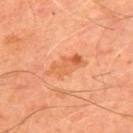Q: Where on the body is the lesion?
A: the upper back
Q: What did automated image analysis measure?
A: an outline eccentricity of about 0.9 (0 = round, 1 = elongated) and a shape-asymmetry score of about 0.3 (0 = symmetric); a mean CIELAB color near L≈63 a*≈29 b*≈43 and a normalized lesion–skin contrast near 6
Q: Who is the patient?
A: male, approximately 50 years of age
Q: What kind of image is this?
A: ~15 mm crop, total-body skin-cancer survey
Q: What is the lesion's diameter?
A: about 4.5 mm
Q: Illumination type?
A: cross-polarized